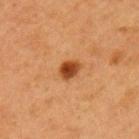site: the left upper arm
image source: total-body-photography crop, ~15 mm field of view
subject: female, roughly 40 years of age
automated metrics: a footprint of about 4.5 mm², an outline eccentricity of about 0.6 (0 = round, 1 = elongated), and a symmetry-axis asymmetry near 0.2; an average lesion color of about L≈40 a*≈25 b*≈37 (CIELAB), about 13 CIELAB-L* units darker than the surrounding skin, and a normalized border contrast of about 10.5; a border-irregularity index near 2/10, a within-lesion color-variation index near 4/10, and peripheral color asymmetry of about 1.5; a detector confidence of about 100 out of 100 that the crop contains a lesion
tile lighting: cross-polarized